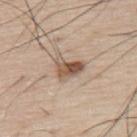Q: Was a biopsy performed?
A: imaged on a skin check; not biopsied
Q: What is the anatomic site?
A: the back
Q: How large is the lesion?
A: about 3.5 mm
Q: What kind of image is this?
A: 15 mm crop, total-body photography
Q: What are the patient's age and sex?
A: male, aged around 75